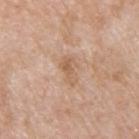{
  "biopsy_status": "not biopsied; imaged during a skin examination",
  "patient": {
    "sex": "male",
    "age_approx": 60
  },
  "automated_metrics": {
    "lesion_detection_confidence_0_100": 100
  },
  "image": {
    "source": "total-body photography crop",
    "field_of_view_mm": 15
  },
  "lighting": "white-light",
  "site": "upper back"
}